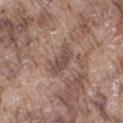Clinical impression: The lesion was tiled from a total-body skin photograph and was not biopsied. Image and clinical context: Approximately 4 mm at its widest. Automated tile analysis of the lesion measured a shape-asymmetry score of about 0.35 (0 = symmetric). The analysis additionally found a border-irregularity index near 4/10, a color-variation rating of about 2.5/10, and peripheral color asymmetry of about 1. Captured under white-light illumination. Cropped from a total-body skin-imaging series; the visible field is about 15 mm. The lesion is located on the right thigh. A male subject in their mid- to late 70s.